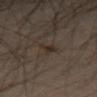The lesion was photographed on a routine skin check and not biopsied; there is no pathology result.
A 15 mm close-up extracted from a 3D total-body photography capture.
The lesion is on the leg.
A male subject, in their mid-60s.
The lesion-visualizer software estimated an average lesion color of about L≈26 a*≈10 b*≈19 (CIELAB) and a normalized border contrast of about 7.5. The software also gave a border-irregularity rating of about 4.5/10, a color-variation rating of about 3/10, and radial color variation of about 1. And it measured an automated nevus-likeness rating near 70 out of 100 and a lesion-detection confidence of about 100/100.
Imaged with cross-polarized lighting.